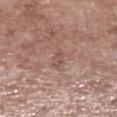notes: catalogued during a skin exam; not biopsied
lighting: white-light
image source: 15 mm crop, total-body photography
automated lesion analysis: an outline eccentricity of about 0.9 (0 = round, 1 = elongated) and two-axis asymmetry of about 0.3
subject: male, in their mid-50s
site: the left lower leg